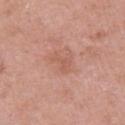Q: Who is the patient?
A: female, in their 50s
Q: What is the anatomic site?
A: the arm
Q: Illumination type?
A: white-light
Q: What did automated image analysis measure?
A: a symmetry-axis asymmetry near 0.5; a border-irregularity index near 5.5/10, a color-variation rating of about 1/10, and a peripheral color-asymmetry measure near 0
Q: Lesion size?
A: about 3.5 mm
Q: What is the imaging modality?
A: ~15 mm tile from a whole-body skin photo